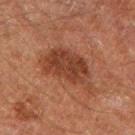The lesion was tiled from a total-body skin photograph and was not biopsied.
The patient is a male in their 60s.
The lesion is on the left thigh.
Cropped from a whole-body photographic skin survey; the tile spans about 15 mm.
Measured at roughly 7 mm in maximum diameter.
This is a cross-polarized tile.
The lesion-visualizer software estimated an area of roughly 18 mm², a shape eccentricity near 0.85, and a symmetry-axis asymmetry near 0.25. And it measured a border-irregularity rating of about 3.5/10, a color-variation rating of about 3.5/10, and radial color variation of about 1. And it measured a nevus-likeness score of about 10/100 and a lesion-detection confidence of about 100/100.